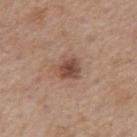No biopsy was performed on this lesion — it was imaged during a full skin examination and was not determined to be concerning.
Automated tile analysis of the lesion measured a lesion area of about 6.5 mm², an eccentricity of roughly 0.45, and a shape-asymmetry score of about 0.25 (0 = symmetric).
The tile uses white-light illumination.
A 15 mm close-up tile from a total-body photography series done for melanoma screening.
The subject is a male aged around 75.
From the right upper arm.
Measured at roughly 3 mm in maximum diameter.